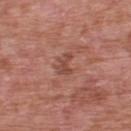  automated_metrics:
    border_irregularity_0_10: 7.0
    color_variation_0_10: 0.5
    nevus_likeness_0_100: 0
    lesion_detection_confidence_0_100: 100
  lighting: white-light
  site: upper back
  image:
    source: total-body photography crop
    field_of_view_mm: 15
  patient:
    sex: male
    age_approx: 70